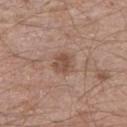Q: Was a biopsy performed?
A: imaged on a skin check; not biopsied
Q: Lesion location?
A: the right upper arm
Q: What is the imaging modality?
A: 15 mm crop, total-body photography
Q: What did automated image analysis measure?
A: a border-irregularity index near 1.5/10, a color-variation rating of about 3/10, and a peripheral color-asymmetry measure near 1; a classifier nevus-likeness of about 5/100 and lesion-presence confidence of about 100/100
Q: Illumination type?
A: white-light illumination
Q: Who is the patient?
A: male, in their 60s
Q: Lesion size?
A: about 2.5 mm